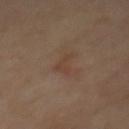Case summary:
- workup · imaged on a skin check; not biopsied
- location · the mid back
- tile lighting · cross-polarized
- lesion diameter · about 3 mm
- automated lesion analysis · an area of roughly 3 mm² and an outline eccentricity of about 0.9 (0 = round, 1 = elongated); a color-variation rating of about 0/10 and a peripheral color-asymmetry measure near 0
- acquisition · total-body-photography crop, ~15 mm field of view